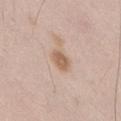Q: Was this lesion biopsied?
A: total-body-photography surveillance lesion; no biopsy
Q: How was the tile lit?
A: white-light
Q: What did automated image analysis measure?
A: a lesion area of about 4 mm², an outline eccentricity of about 0.75 (0 = round, 1 = elongated), and a symmetry-axis asymmetry near 0.25; roughly 11 lightness units darker than nearby skin and a normalized border contrast of about 8; a nevus-likeness score of about 90/100 and a detector confidence of about 100 out of 100 that the crop contains a lesion
Q: What is the anatomic site?
A: the lower back
Q: What is the lesion's diameter?
A: ≈3 mm
Q: What kind of image is this?
A: total-body-photography crop, ~15 mm field of view
Q: What are the patient's age and sex?
A: male, about 50 years old